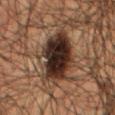Image and clinical context:
This image is a 15 mm lesion crop taken from a total-body photograph. The subject is a male roughly 50 years of age. From the mid back.